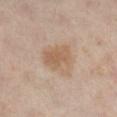Case summary:
* biopsy status · imaged on a skin check; not biopsied
* body site · the left leg
* imaging modality · 15 mm crop, total-body photography
* patient · female, aged 38–42
* automated metrics · border irregularity of about 2.5 on a 0–10 scale and a color-variation rating of about 3.5/10; lesion-presence confidence of about 100/100
* lighting · cross-polarized illumination
* lesion diameter · ≈4.5 mm